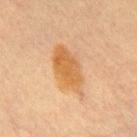biopsy status=no biopsy performed (imaged during a skin exam)
size=~6 mm (longest diameter)
tile lighting=cross-polarized
image source=~15 mm tile from a whole-body skin photo
body site=the front of the torso
automated metrics=an area of roughly 13 mm² and a shape eccentricity near 0.85; a nevus-likeness score of about 95/100 and a lesion-detection confidence of about 100/100
subject=female, aged 58–62